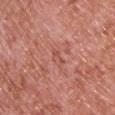| field | value |
|---|---|
| biopsy status | catalogued during a skin exam; not biopsied |
| patient | male, aged around 70 |
| lesion diameter | about 2.5 mm |
| lighting | white-light |
| image source | ~15 mm tile from a whole-body skin photo |
| TBP lesion metrics | a mean CIELAB color near L≈52 a*≈29 b*≈28, about 7 CIELAB-L* units darker than the surrounding skin, and a lesion-to-skin contrast of about 5 (normalized; higher = more distinct); internal color variation of about 0 on a 0–10 scale and radial color variation of about 0; a detector confidence of about 95 out of 100 that the crop contains a lesion |
| location | the back |